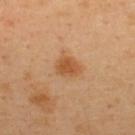• workup — imaged on a skin check; not biopsied
• lesion size — about 3 mm
• site — the upper back
• patient — male, aged 38–42
• lighting — cross-polarized illumination
• image source — 15 mm crop, total-body photography